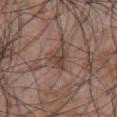<record>
<biopsy_status>not biopsied; imaged during a skin examination</biopsy_status>
<lighting>white-light</lighting>
<image>
  <source>total-body photography crop</source>
  <field_of_view_mm>15</field_of_view_mm>
</image>
<site>chest</site>
<lesion_size>
  <long_diameter_mm_approx>3.0</long_diameter_mm_approx>
</lesion_size>
<patient>
  <sex>male</sex>
  <age_approx>70</age_approx>
</patient>
</record>